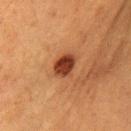Captured during whole-body skin photography for melanoma surveillance; the lesion was not biopsied. A lesion tile, about 15 mm wide, cut from a 3D total-body photograph. The patient is a female roughly 50 years of age. Captured under cross-polarized illumination. An algorithmic analysis of the crop reported an outline eccentricity of about 0.7 (0 = round, 1 = elongated). And it measured a border-irregularity rating of about 2/10 and radial color variation of about 1.5. Located on the chest.